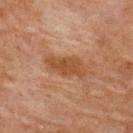The lesion was tiled from a total-body skin photograph and was not biopsied. The subject is a female aged approximately 80. The lesion's longest dimension is about 5 mm. Imaged with cross-polarized lighting. Cropped from a total-body skin-imaging series; the visible field is about 15 mm. On the upper back.